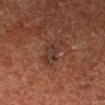Recorded during total-body skin imaging; not selected for excision or biopsy.
Approximately 4 mm at its widest.
A 15 mm crop from a total-body photograph taken for skin-cancer surveillance.
On the leg.
A male patient, in their mid- to late 60s.
Automated image analysis of the tile measured roughly 6 lightness units darker than nearby skin and a lesion-to-skin contrast of about 6.5 (normalized; higher = more distinct). And it measured border irregularity of about 4.5 on a 0–10 scale, a color-variation rating of about 3/10, and peripheral color asymmetry of about 1.
This is a cross-polarized tile.